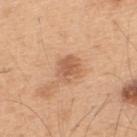Imaged during a routine full-body skin examination; the lesion was not biopsied and no histopathology is available.